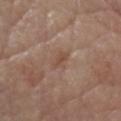Part of a total-body skin-imaging series; this lesion was reviewed on a skin check and was not flagged for biopsy.
The patient is a male aged approximately 80.
About 3.5 mm across.
The tile uses white-light illumination.
On the head or neck.
The lesion-visualizer software estimated a mean CIELAB color near L≈48 a*≈18 b*≈27, about 7 CIELAB-L* units darker than the surrounding skin, and a normalized border contrast of about 5.5. It also reported border irregularity of about 4.5 on a 0–10 scale, a color-variation rating of about 0/10, and radial color variation of about 0. It also reported a classifier nevus-likeness of about 0/100 and a lesion-detection confidence of about 90/100.
Cropped from a whole-body photographic skin survey; the tile spans about 15 mm.